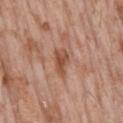This lesion was catalogued during total-body skin photography and was not selected for biopsy. Captured under white-light illumination. A roughly 15 mm field-of-view crop from a total-body skin photograph. A male patient aged 73 to 77. Measured at roughly 3.5 mm in maximum diameter. On the back.